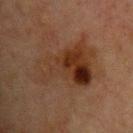Impression:
The lesion was tiled from a total-body skin photograph and was not biopsied.
Acquisition and patient details:
Approximately 8.5 mm at its widest. An algorithmic analysis of the crop reported an average lesion color of about L≈26 a*≈16 b*≈24 (CIELAB), roughly 7 lightness units darker than nearby skin, and a normalized border contrast of about 8. It also reported border irregularity of about 6 on a 0–10 scale and a color-variation rating of about 9.5/10. The analysis additionally found a classifier nevus-likeness of about 35/100 and lesion-presence confidence of about 100/100. This is a cross-polarized tile. A male subject aged around 65. Located on the chest. This image is a 15 mm lesion crop taken from a total-body photograph.